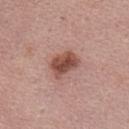follow-up: no biopsy performed (imaged during a skin exam); subject: female, aged 48 to 52; acquisition: ~15 mm crop, total-body skin-cancer survey; location: the abdomen.